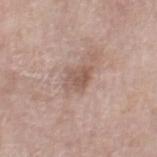* follow-up: imaged on a skin check; not biopsied
* image source: 15 mm crop, total-body photography
* location: the right thigh
* TBP lesion metrics: border irregularity of about 3 on a 0–10 scale and a peripheral color-asymmetry measure near 1
* lesion diameter: about 3 mm
* tile lighting: white-light illumination
* subject: female, in their mid- to late 70s